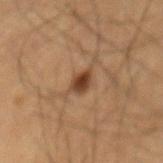A male patient, about 60 years old. A 15 mm close-up extracted from a 3D total-body photography capture. Measured at roughly 3 mm in maximum diameter. This is a cross-polarized tile.